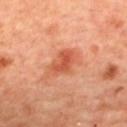Clinical impression:
Imaged during a routine full-body skin examination; the lesion was not biopsied and no histopathology is available.
Clinical summary:
The lesion is on the upper back. The subject is a female in their mid- to late 40s. A roughly 15 mm field-of-view crop from a total-body skin photograph. Imaged with cross-polarized lighting. The recorded lesion diameter is about 4 mm. Automated image analysis of the tile measured an average lesion color of about L≈55 a*≈32 b*≈37 (CIELAB), about 10 CIELAB-L* units darker than the surrounding skin, and a normalized lesion–skin contrast near 7.